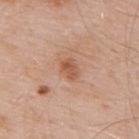Q: Was a biopsy performed?
A: no biopsy performed (imaged during a skin exam)
Q: What did automated image analysis measure?
A: a mean CIELAB color near L≈55 a*≈23 b*≈33 and a normalized border contrast of about 6.5; a border-irregularity index near 2.5/10, a within-lesion color-variation index near 3/10, and a peripheral color-asymmetry measure near 1
Q: What is the anatomic site?
A: the upper back
Q: What are the patient's age and sex?
A: male, aged 53 to 57
Q: How large is the lesion?
A: about 2.5 mm
Q: What kind of image is this?
A: total-body-photography crop, ~15 mm field of view